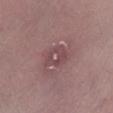workup: catalogued during a skin exam; not biopsied
patient: female, aged 43 to 47
imaging modality: ~15 mm crop, total-body skin-cancer survey
location: the left lower leg
TBP lesion metrics: a mean CIELAB color near L≈46 a*≈21 b*≈16, roughly 7 lightness units darker than nearby skin, and a lesion-to-skin contrast of about 5.5 (normalized; higher = more distinct); a within-lesion color-variation index near 6/10 and peripheral color asymmetry of about 2; an automated nevus-likeness rating near 0 out of 100 and a lesion-detection confidence of about 90/100